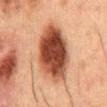Assessment: Imaged during a routine full-body skin examination; the lesion was not biopsied and no histopathology is available. Clinical summary: The patient is a male aged approximately 50. The total-body-photography lesion software estimated a lesion area of about 28 mm² and a shape eccentricity near 0.85. It also reported a mean CIELAB color near L≈37 a*≈21 b*≈27 and roughly 17 lightness units darker than nearby skin. The analysis additionally found an automated nevus-likeness rating near 100 out of 100 and a lesion-detection confidence of about 100/100. The lesion is located on the mid back. The tile uses cross-polarized illumination. About 8.5 mm across. A 15 mm close-up extracted from a 3D total-body photography capture.